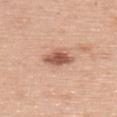Findings:
• biopsy status: catalogued during a skin exam; not biopsied
• image source: 15 mm crop, total-body photography
• subject: female, about 60 years old
• site: the upper back